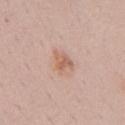biopsy status = catalogued during a skin exam; not biopsied | tile lighting = white-light illumination | image source = 15 mm crop, total-body photography | anatomic site = the chest | subject = male, in their 50s.